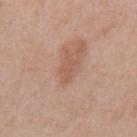follow-up=catalogued during a skin exam; not biopsied
automated lesion analysis=border irregularity of about 9.5 on a 0–10 scale and a color-variation rating of about 0/10; an automated nevus-likeness rating near 0 out of 100 and a lesion-detection confidence of about 100/100
image=~15 mm crop, total-body skin-cancer survey
anatomic site=the arm
tile lighting=white-light illumination
subject=female, aged 38 to 42